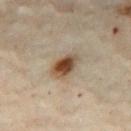Clinical impression:
Captured during whole-body skin photography for melanoma surveillance; the lesion was not biopsied.
Image and clinical context:
From the leg. The lesion's longest dimension is about 4 mm. A close-up tile cropped from a whole-body skin photograph, about 15 mm across. Imaged with cross-polarized lighting. Automated image analysis of the tile measured a color-variation rating of about 8/10 and peripheral color asymmetry of about 2. The software also gave an automated nevus-likeness rating near 100 out of 100 and a lesion-detection confidence of about 100/100. The subject is a female aged 58–62.